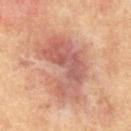Impression:
Recorded during total-body skin imaging; not selected for excision or biopsy.